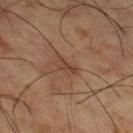Part of a total-body skin-imaging series; this lesion was reviewed on a skin check and was not flagged for biopsy.
The lesion's longest dimension is about 2.5 mm.
The lesion-visualizer software estimated a lesion color around L≈41 a*≈19 b*≈27 in CIELAB and a normalized lesion–skin contrast near 6.5.
This is a cross-polarized tile.
On the right thigh.
The subject is a male aged approximately 65.
A 15 mm close-up extracted from a 3D total-body photography capture.